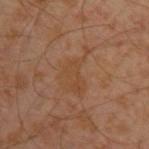image source: ~15 mm crop, total-body skin-cancer survey; subject: male, roughly 30 years of age; site: the arm; illumination: cross-polarized.